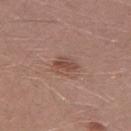workup: catalogued during a skin exam; not biopsied | acquisition: 15 mm crop, total-body photography | body site: the left thigh | image-analysis metrics: a footprint of about 4.5 mm², an outline eccentricity of about 0.7 (0 = round, 1 = elongated), and a shape-asymmetry score of about 0.2 (0 = symmetric); a lesion color around L≈48 a*≈21 b*≈26 in CIELAB, about 9 CIELAB-L* units darker than the surrounding skin, and a lesion-to-skin contrast of about 7 (normalized; higher = more distinct) | patient: male, aged 28 to 32 | lighting: white-light illumination | lesion size: ≈3 mm.